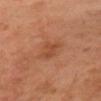• notes · no biopsy performed (imaged during a skin exam)
• subject · female, aged around 55
• image source · total-body-photography crop, ~15 mm field of view
• location · the arm